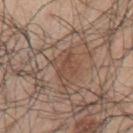<lesion>
  <lighting>white-light</lighting>
  <patient>
    <sex>male</sex>
    <age_approx>70</age_approx>
  </patient>
  <site>arm</site>
  <image>
    <source>total-body photography crop</source>
    <field_of_view_mm>15</field_of_view_mm>
  </image>
</lesion>